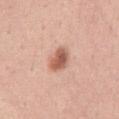<case>
  <biopsy_status>not biopsied; imaged during a skin examination</biopsy_status>
  <automated_metrics>
    <border_irregularity_0_10>2.0</border_irregularity_0_10>
    <color_variation_0_10>4.0</color_variation_0_10>
    <nevus_likeness_0_100>95</nevus_likeness_0_100>
  </automated_metrics>
  <image>
    <source>total-body photography crop</source>
    <field_of_view_mm>15</field_of_view_mm>
  </image>
  <patient>
    <sex>female</sex>
    <age_approx>35</age_approx>
  </patient>
  <site>chest</site>
</case>